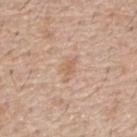{
  "biopsy_status": "not biopsied; imaged during a skin examination",
  "image": {
    "source": "total-body photography crop",
    "field_of_view_mm": 15
  },
  "automated_metrics": {
    "lesion_detection_confidence_0_100": 100
  },
  "site": "back",
  "patient": {
    "sex": "male",
    "age_approx": 55
  },
  "lesion_size": {
    "long_diameter_mm_approx": 2.5
  },
  "lighting": "white-light"
}